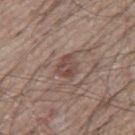biopsy status = catalogued during a skin exam; not biopsied
image-analysis metrics = a footprint of about 5.5 mm² and a symmetry-axis asymmetry near 0.25; an average lesion color of about L≈46 a*≈17 b*≈23 (CIELAB) and about 8 CIELAB-L* units darker than the surrounding skin; a border-irregularity rating of about 2.5/10, a within-lesion color-variation index near 4/10, and a peripheral color-asymmetry measure near 1.5; an automated nevus-likeness rating near 55 out of 100
location = the mid back
lesion size = ≈3 mm
tile lighting = white-light
imaging modality = 15 mm crop, total-body photography
subject = male, in their mid-60s